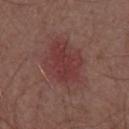<tbp_lesion>
<biopsy_status>not biopsied; imaged during a skin examination</biopsy_status>
<patient>
  <sex>male</sex>
  <age_approx>55</age_approx>
</patient>
<automated_metrics>
  <area_mm2_approx>23.0</area_mm2_approx>
  <eccentricity>0.65</eccentricity>
  <shape_asymmetry>0.2</shape_asymmetry>
  <cielab_L>38</cielab_L>
  <cielab_a>24</cielab_a>
  <cielab_b>21</cielab_b>
  <vs_skin_darker_L>7.0</vs_skin_darker_L>
  <vs_skin_contrast_norm>6.0</vs_skin_contrast_norm>
</automated_metrics>
<lesion_size>
  <long_diameter_mm_approx>6.5</long_diameter_mm_approx>
</lesion_size>
<image>
  <source>total-body photography crop</source>
  <field_of_view_mm>15</field_of_view_mm>
</image>
<lighting>white-light</lighting>
<site>chest</site>
</tbp_lesion>